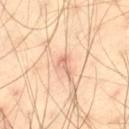workup — catalogued during a skin exam; not biopsied | subject — male, aged 38 to 42 | image source — 15 mm crop, total-body photography | lighting — cross-polarized | lesion size — ~2.5 mm (longest diameter) | body site — the leg.